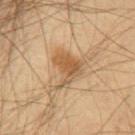Assessment:
The lesion was photographed on a routine skin check and not biopsied; there is no pathology result.
Image and clinical context:
Approximately 4.5 mm at its widest. The total-body-photography lesion software estimated an eccentricity of roughly 0.6 and two-axis asymmetry of about 0.5. And it measured an average lesion color of about L≈53 a*≈17 b*≈35 (CIELAB), roughly 10 lightness units darker than nearby skin, and a lesion-to-skin contrast of about 7.5 (normalized; higher = more distinct). The software also gave a classifier nevus-likeness of about 55/100 and a lesion-detection confidence of about 100/100. Imaged with cross-polarized lighting. The lesion is on the chest. The subject is a male approximately 55 years of age. A close-up tile cropped from a whole-body skin photograph, about 15 mm across.